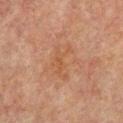The lesion was tiled from a total-body skin photograph and was not biopsied.
An algorithmic analysis of the crop reported border irregularity of about 5.5 on a 0–10 scale, a color-variation rating of about 3/10, and peripheral color asymmetry of about 1.
The lesion is on the back.
A 15 mm close-up extracted from a 3D total-body photography capture.
The recorded lesion diameter is about 4.5 mm.
A male subject, approximately 65 years of age.